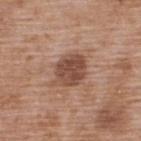Clinical impression:
Captured during whole-body skin photography for melanoma surveillance; the lesion was not biopsied.
Acquisition and patient details:
The total-body-photography lesion software estimated a border-irregularity index near 2/10, a color-variation rating of about 4/10, and a peripheral color-asymmetry measure near 1.5. It also reported a nevus-likeness score of about 45/100 and a lesion-detection confidence of about 100/100. The recorded lesion diameter is about 4.5 mm. The patient is a male aged around 50. From the upper back. This is a white-light tile. A 15 mm close-up tile from a total-body photography series done for melanoma screening.